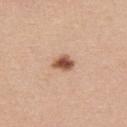| feature | finding |
|---|---|
| subject | female, about 45 years old |
| anatomic site | the chest |
| acquisition | total-body-photography crop, ~15 mm field of view |